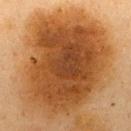notes: imaged on a skin check; not biopsied
imaging modality: ~15 mm crop, total-body skin-cancer survey
illumination: cross-polarized illumination
site: the upper back
lesion diameter: ≈11.5 mm
automated lesion analysis: border irregularity of about 2.5 on a 0–10 scale; a classifier nevus-likeness of about 100/100 and a lesion-detection confidence of about 100/100
subject: female, approximately 40 years of age